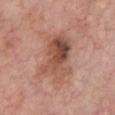<record>
<biopsy_status>not biopsied; imaged during a skin examination</biopsy_status>
<lesion_size>
  <long_diameter_mm_approx>6.5</long_diameter_mm_approx>
</lesion_size>
<lighting>white-light</lighting>
<patient>
  <sex>male</sex>
  <age_approx>60</age_approx>
</patient>
<automated_metrics>
  <cielab_L>51</cielab_L>
  <cielab_a>22</cielab_a>
  <cielab_b>29</cielab_b>
  <vs_skin_darker_L>11.0</vs_skin_darker_L>
  <vs_skin_contrast_norm>8.0</vs_skin_contrast_norm>
  <border_irregularity_0_10>4.0</border_irregularity_0_10>
  <color_variation_0_10>9.5</color_variation_0_10>
  <peripheral_color_asymmetry>4.0</peripheral_color_asymmetry>
</automated_metrics>
<image>
  <source>total-body photography crop</source>
  <field_of_view_mm>15</field_of_view_mm>
</image>
<site>chest</site>
</record>